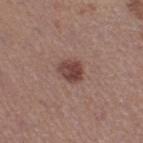Q: Was a biopsy performed?
A: imaged on a skin check; not biopsied
Q: What kind of image is this?
A: ~15 mm tile from a whole-body skin photo
Q: Who is the patient?
A: female, aged approximately 35
Q: Where on the body is the lesion?
A: the right thigh
Q: Lesion size?
A: ~3 mm (longest diameter)
Q: What lighting was used for the tile?
A: white-light illumination
Q: What did automated image analysis measure?
A: a footprint of about 6 mm², an outline eccentricity of about 0.55 (0 = round, 1 = elongated), and a symmetry-axis asymmetry near 0.2